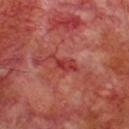Q: Was a biopsy performed?
A: total-body-photography surveillance lesion; no biopsy
Q: Patient demographics?
A: male, roughly 70 years of age
Q: What kind of image is this?
A: 15 mm crop, total-body photography
Q: What is the anatomic site?
A: the chest
Q: Lesion size?
A: ~3.5 mm (longest diameter)